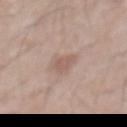  biopsy_status: not biopsied; imaged during a skin examination
  automated_metrics:
    area_mm2_approx: 4.5
    eccentricity: 0.6
    shape_asymmetry: 0.35
    cielab_L: 57
    cielab_a: 17
    cielab_b: 25
    vs_skin_darker_L: 8.0
    border_irregularity_0_10: 3.0
    color_variation_0_10: 1.0
    nevus_likeness_0_100: 5
    lesion_detection_confidence_0_100: 100
  patient:
    sex: male
    age_approx: 70
  site: front of the torso
  image:
    source: total-body photography crop
    field_of_view_mm: 15
  lighting: white-light
  lesion_size:
    long_diameter_mm_approx: 2.5